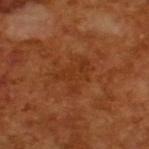Case summary:
– workup · no biopsy performed (imaged during a skin exam)
– illumination · cross-polarized
– subject · male, approximately 65 years of age
– image-analysis metrics · a footprint of about 9 mm² and an outline eccentricity of about 0.7 (0 = round, 1 = elongated); an average lesion color of about L≈34 a*≈25 b*≈35 (CIELAB), a lesion–skin lightness drop of about 5, and a normalized lesion–skin contrast near 5; a border-irregularity rating of about 6.5/10; a nevus-likeness score of about 0/100 and a detector confidence of about 100 out of 100 that the crop contains a lesion
– image · total-body-photography crop, ~15 mm field of view
– lesion diameter · about 4 mm